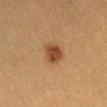Q: Was a biopsy performed?
A: imaged on a skin check; not biopsied
Q: How was this image acquired?
A: ~15 mm crop, total-body skin-cancer survey
Q: Patient demographics?
A: female, aged around 40
Q: Lesion size?
A: about 2.5 mm
Q: Lesion location?
A: the abdomen